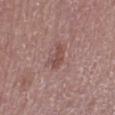follow-up = total-body-photography surveillance lesion; no biopsy | imaging modality = ~15 mm crop, total-body skin-cancer survey | patient = male, approximately 75 years of age | lesion size = about 2.5 mm | location = the left lower leg | lighting = white-light | automated metrics = border irregularity of about 4 on a 0–10 scale and a peripheral color-asymmetry measure near 0.5; a nevus-likeness score of about 0/100.